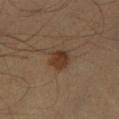biopsy status: catalogued during a skin exam; not biopsied | lighting: cross-polarized illumination | size: ~3 mm (longest diameter) | image source: 15 mm crop, total-body photography | TBP lesion metrics: an average lesion color of about L≈36 a*≈18 b*≈28 (CIELAB) and a lesion-to-skin contrast of about 8.5 (normalized; higher = more distinct); a border-irregularity rating of about 1.5/10 and internal color variation of about 3 on a 0–10 scale | location: the leg | subject: male, about 40 years old.